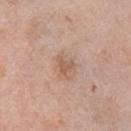This lesion was catalogued during total-body skin photography and was not selected for biopsy.
The total-body-photography lesion software estimated an area of roughly 3.5 mm² and an eccentricity of roughly 0.65.
A female subject, about 65 years old.
Approximately 2.5 mm at its widest.
Located on the right upper arm.
A close-up tile cropped from a whole-body skin photograph, about 15 mm across.
This is a white-light tile.